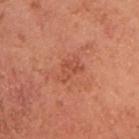Image and clinical context: The lesion is on the head or neck. A roughly 15 mm field-of-view crop from a total-body skin photograph. The lesion's longest dimension is about 3 mm. Imaged with cross-polarized lighting. A female subject aged approximately 55.